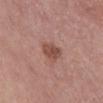Clinical impression:
No biopsy was performed on this lesion — it was imaged during a full skin examination and was not determined to be concerning.
Context:
A male subject, approximately 75 years of age. Cropped from a whole-body photographic skin survey; the tile spans about 15 mm. An algorithmic analysis of the crop reported roughly 10 lightness units darker than nearby skin and a lesion-to-skin contrast of about 7.5 (normalized; higher = more distinct). It also reported a border-irregularity index near 2/10 and a within-lesion color-variation index near 3/10. The recorded lesion diameter is about 3 mm. This is a white-light tile. Located on the abdomen.